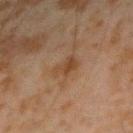workup = total-body-photography surveillance lesion; no biopsy | site = the right forearm | diameter = ≈3 mm | subject = male, in their mid-40s | image = 15 mm crop, total-body photography | automated metrics = a mean CIELAB color near L≈36 a*≈16 b*≈27, about 6 CIELAB-L* units darker than the surrounding skin, and a normalized lesion–skin contrast near 6; an automated nevus-likeness rating near 5 out of 100 and a detector confidence of about 100 out of 100 that the crop contains a lesion.